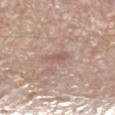Case summary:
• biopsy status — imaged on a skin check; not biopsied
• patient — male, in their 70s
• site — the left lower leg
• tile lighting — white-light illumination
• image source — 15 mm crop, total-body photography